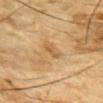Notes:
- notes: imaged on a skin check; not biopsied
- image source: ~15 mm tile from a whole-body skin photo
- lesion diameter: ≈2.5 mm
- patient: male, aged 83 or over
- anatomic site: the chest